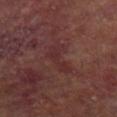This lesion was catalogued during total-body skin photography and was not selected for biopsy.
The lesion is on the left lower leg.
Captured under cross-polarized illumination.
Automated image analysis of the tile measured a color-variation rating of about 3/10 and peripheral color asymmetry of about 1.
A 15 mm close-up tile from a total-body photography series done for melanoma screening.
Approximately 5 mm at its widest.
The subject is a male aged 63–67.